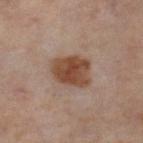The lesion was tiled from a total-body skin photograph and was not biopsied.
The recorded lesion diameter is about 5 mm.
The lesion is on the left thigh.
The tile uses cross-polarized illumination.
This image is a 15 mm lesion crop taken from a total-body photograph.
A female subject, aged approximately 50.
The total-body-photography lesion software estimated an area of roughly 13 mm² and a shape eccentricity near 0.65. The analysis additionally found a border-irregularity rating of about 2.5/10, a color-variation rating of about 3.5/10, and peripheral color asymmetry of about 1. And it measured a classifier nevus-likeness of about 95/100 and a detector confidence of about 100 out of 100 that the crop contains a lesion.